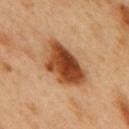Imaged during a routine full-body skin examination; the lesion was not biopsied and no histopathology is available. Automated image analysis of the tile measured a color-variation rating of about 8.5/10 and a peripheral color-asymmetry measure near 3. A 15 mm crop from a total-body photograph taken for skin-cancer surveillance. Imaged with cross-polarized lighting. The subject is a male about 50 years old. Located on the mid back.